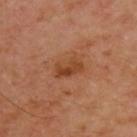Captured during whole-body skin photography for melanoma surveillance; the lesion was not biopsied.